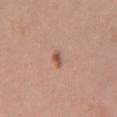Part of a total-body skin-imaging series; this lesion was reviewed on a skin check and was not flagged for biopsy. The patient is a female roughly 40 years of age. A region of skin cropped from a whole-body photographic capture, roughly 15 mm wide. The tile uses white-light illumination. Approximately 3 mm at its widest. On the mid back.